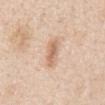workup: catalogued during a skin exam; not biopsied | imaging modality: total-body-photography crop, ~15 mm field of view | diameter: ~3.5 mm (longest diameter) | site: the abdomen | lighting: white-light | patient: male, aged around 60 | automated lesion analysis: a lesion area of about 6 mm², an outline eccentricity of about 0.9 (0 = round, 1 = elongated), and a symmetry-axis asymmetry near 0.2; a mean CIELAB color near L≈66 a*≈19 b*≈32, about 11 CIELAB-L* units darker than the surrounding skin, and a lesion-to-skin contrast of about 7 (normalized; higher = more distinct).